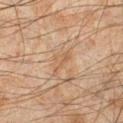The lesion was photographed on a routine skin check and not biopsied; there is no pathology result.
Longest diameter approximately 3.5 mm.
This is a cross-polarized tile.
From the left lower leg.
The subject is a male roughly 45 years of age.
An algorithmic analysis of the crop reported a nevus-likeness score of about 0/100 and a lesion-detection confidence of about 60/100.
A 15 mm crop from a total-body photograph taken for skin-cancer surveillance.